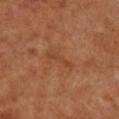Impression:
No biopsy was performed on this lesion — it was imaged during a full skin examination and was not determined to be concerning.
Image and clinical context:
About 4 mm across. The lesion is located on the right forearm. Automated image analysis of the tile measured a mean CIELAB color near L≈43 a*≈24 b*≈34, about 6 CIELAB-L* units darker than the surrounding skin, and a lesion-to-skin contrast of about 5 (normalized; higher = more distinct). And it measured a border-irregularity index near 4.5/10, a within-lesion color-variation index near 0.5/10, and a peripheral color-asymmetry measure near 0. A male patient, aged approximately 55. The tile uses cross-polarized illumination. Cropped from a whole-body photographic skin survey; the tile spans about 15 mm.